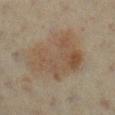Acquisition and patient details: Measured at roughly 7.5 mm in maximum diameter. From the right lower leg. A roughly 15 mm field-of-view crop from a total-body skin photograph. The tile uses cross-polarized illumination. A female patient aged 63–67.